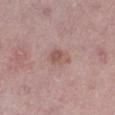Q: Was a biopsy performed?
A: total-body-photography surveillance lesion; no biopsy
Q: What are the patient's age and sex?
A: female, aged approximately 50
Q: What is the lesion's diameter?
A: ≈3 mm
Q: How was this image acquired?
A: ~15 mm crop, total-body skin-cancer survey
Q: What is the anatomic site?
A: the leg
Q: What lighting was used for the tile?
A: white-light